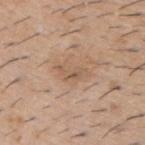{"biopsy_status": "not biopsied; imaged during a skin examination", "lighting": "white-light", "lesion_size": {"long_diameter_mm_approx": 4.0}, "site": "upper back", "patient": {"sex": "male", "age_approx": 60}, "image": {"source": "total-body photography crop", "field_of_view_mm": 15}}